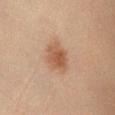biopsy status: no biopsy performed (imaged during a skin exam)
site: the right lower leg
TBP lesion metrics: a lesion color around L≈49 a*≈20 b*≈30 in CIELAB, roughly 9 lightness units darker than nearby skin, and a lesion-to-skin contrast of about 7.5 (normalized; higher = more distinct); border irregularity of about 2 on a 0–10 scale, a within-lesion color-variation index near 3.5/10, and peripheral color asymmetry of about 1
image: total-body-photography crop, ~15 mm field of view
tile lighting: cross-polarized illumination
patient: female, aged 28–32